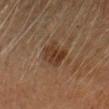A 15 mm close-up extracted from a 3D total-body photography capture.
A female subject aged 38 to 42.
Located on the head or neck.
The lesion-visualizer software estimated a lesion area of about 8 mm². The analysis additionally found a detector confidence of about 100 out of 100 that the crop contains a lesion.